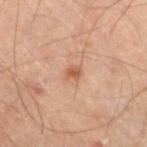The lesion was photographed on a routine skin check and not biopsied; there is no pathology result. The total-body-photography lesion software estimated a shape eccentricity near 0.6 and a shape-asymmetry score of about 0.25 (0 = symmetric). The analysis additionally found a mean CIELAB color near L≈56 a*≈22 b*≈34, a lesion–skin lightness drop of about 9, and a normalized border contrast of about 7. The software also gave a border-irregularity index near 2/10, a color-variation rating of about 2/10, and radial color variation of about 0.5. A 15 mm crop from a total-body photograph taken for skin-cancer surveillance. The lesion is located on the right thigh. A male subject in their 70s. The recorded lesion diameter is about 2 mm.